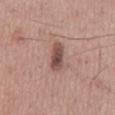Captured during whole-body skin photography for melanoma surveillance; the lesion was not biopsied. The lesion's longest dimension is about 4 mm. A lesion tile, about 15 mm wide, cut from a 3D total-body photograph. From the mid back. The subject is a male aged around 55.